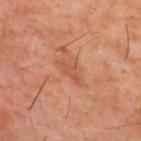site: mid back
patient:
  sex: male
  age_approx: 60
image:
  source: total-body photography crop
  field_of_view_mm: 15
lighting: cross-polarized